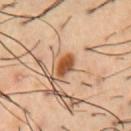<lesion>
  <biopsy_status>not biopsied; imaged during a skin examination</biopsy_status>
  <patient>
    <sex>male</sex>
    <age_approx>55</age_approx>
  </patient>
  <automated_metrics>
    <area_mm2_approx>4.5</area_mm2_approx>
    <eccentricity>0.8</eccentricity>
    <shape_asymmetry>0.2</shape_asymmetry>
    <cielab_L>50</cielab_L>
    <cielab_a>24</cielab_a>
    <cielab_b>37</cielab_b>
    <vs_skin_darker_L>16.0</vs_skin_darker_L>
    <vs_skin_contrast_norm>11.5</vs_skin_contrast_norm>
    <border_irregularity_0_10>2.0</border_irregularity_0_10>
    <color_variation_0_10>4.5</color_variation_0_10>
    <peripheral_color_asymmetry>1.5</peripheral_color_asymmetry>
    <lesion_detection_confidence_0_100>100</lesion_detection_confidence_0_100>
  </automated_metrics>
  <image>
    <source>total-body photography crop</source>
    <field_of_view_mm>15</field_of_view_mm>
  </image>
  <site>chest</site>
  <lighting>cross-polarized</lighting>
</lesion>